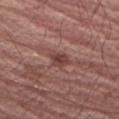notes: catalogued during a skin exam; not biopsied
TBP lesion metrics: a shape eccentricity near 0.7; an average lesion color of about L≈41 a*≈23 b*≈23 (CIELAB) and roughly 9 lightness units darker than nearby skin; a border-irregularity rating of about 2.5/10 and a color-variation rating of about 2/10; a nevus-likeness score of about 15/100 and lesion-presence confidence of about 100/100
subject: male, roughly 65 years of age
imaging modality: ~15 mm tile from a whole-body skin photo
tile lighting: white-light illumination
diameter: about 2.5 mm
anatomic site: the right upper arm